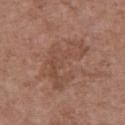Recorded during total-body skin imaging; not selected for excision or biopsy. The lesion is on the chest. A female subject aged 73 to 77. A 15 mm close-up extracted from a 3D total-body photography capture.